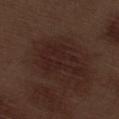workup — total-body-photography surveillance lesion; no biopsy
patient — male, aged 68 to 72
location — the right thigh
acquisition — 15 mm crop, total-body photography